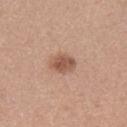Notes:
• biopsy status · no biopsy performed (imaged during a skin exam)
• TBP lesion metrics · an eccentricity of roughly 0.65 and a symmetry-axis asymmetry near 0.15; internal color variation of about 3.5 on a 0–10 scale
• subject · female, in their mid- to late 20s
• lesion size · ~3 mm (longest diameter)
• image · ~15 mm crop, total-body skin-cancer survey
• site · the upper back
• illumination · white-light